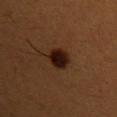Clinical impression: Recorded during total-body skin imaging; not selected for excision or biopsy. Background: From the left upper arm. A female patient aged 38 to 42. A roughly 15 mm field-of-view crop from a total-body skin photograph.